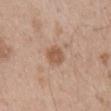Q: Is there a histopathology result?
A: total-body-photography surveillance lesion; no biopsy
Q: Lesion size?
A: ~2.5 mm (longest diameter)
Q: What did automated image analysis measure?
A: a shape eccentricity near 0.4 and a shape-asymmetry score of about 0.2 (0 = symmetric); a classifier nevus-likeness of about 55/100 and a detector confidence of about 100 out of 100 that the crop contains a lesion
Q: Who is the patient?
A: male, aged around 50
Q: Lesion location?
A: the mid back
Q: What kind of image is this?
A: ~15 mm tile from a whole-body skin photo
Q: What lighting was used for the tile?
A: white-light illumination